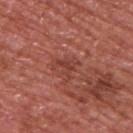The patient is a male aged approximately 65.
From the upper back.
Cropped from a whole-body photographic skin survey; the tile spans about 15 mm.